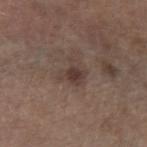Part of a total-body skin-imaging series; this lesion was reviewed on a skin check and was not flagged for biopsy. A male subject aged approximately 70. Imaged with white-light lighting. Cropped from a total-body skin-imaging series; the visible field is about 15 mm. Measured at roughly 3 mm in maximum diameter. On the left forearm.